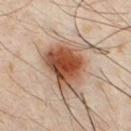workup: catalogued during a skin exam; not biopsied
imaging modality: total-body-photography crop, ~15 mm field of view
site: the chest
size: about 6.5 mm
patient: male, in their mid- to late 30s
automated metrics: an area of roughly 21 mm², an outline eccentricity of about 0.7 (0 = round, 1 = elongated), and a symmetry-axis asymmetry near 0.25; an automated nevus-likeness rating near 100 out of 100 and a detector confidence of about 100 out of 100 that the crop contains a lesion